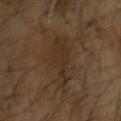Imaged during a routine full-body skin examination; the lesion was not biopsied and no histopathology is available. A male subject aged 53–57. Longest diameter approximately 6 mm. Cropped from a whole-body photographic skin survey; the tile spans about 15 mm. Captured under cross-polarized illumination. Located on the head or neck.